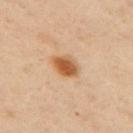biopsy status: total-body-photography surveillance lesion; no biopsy
imaging modality: ~15 mm crop, total-body skin-cancer survey
TBP lesion metrics: two-axis asymmetry of about 0.15; an average lesion color of about L≈58 a*≈23 b*≈41 (CIELAB) and about 14 CIELAB-L* units darker than the surrounding skin; border irregularity of about 1.5 on a 0–10 scale and radial color variation of about 1.5; a classifier nevus-likeness of about 100/100
patient: female, aged around 40
lesion size: about 3 mm
location: the left arm